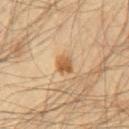Part of a total-body skin-imaging series; this lesion was reviewed on a skin check and was not flagged for biopsy. The subject is a male in their mid-50s. From the chest. The lesion-visualizer software estimated a lesion area of about 3.5 mm² and a shape-asymmetry score of about 0.2 (0 = symmetric). The software also gave a mean CIELAB color near L≈55 a*≈19 b*≈38 and a normalized border contrast of about 8.5. A 15 mm close-up extracted from a 3D total-body photography capture.